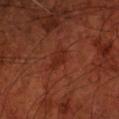workup: catalogued during a skin exam; not biopsied | image source: ~15 mm tile from a whole-body skin photo | location: the front of the torso | patient: male, aged around 70.